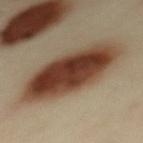image: ~15 mm tile from a whole-body skin photo | tile lighting: cross-polarized | TBP lesion metrics: a footprint of about 38 mm², an eccentricity of roughly 0.9, and a shape-asymmetry score of about 0.1 (0 = symmetric); border irregularity of about 2 on a 0–10 scale, internal color variation of about 8.5 on a 0–10 scale, and radial color variation of about 3; a classifier nevus-likeness of about 100/100 | location: the upper back | subject: female, aged 38 to 42 | lesion size: ≈10 mm.